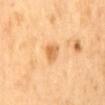Recorded during total-body skin imaging; not selected for excision or biopsy. A male patient in their mid- to late 60s. The lesion is on the mid back. About 2.5 mm across. Cropped from a whole-body photographic skin survey; the tile spans about 15 mm. The tile uses cross-polarized illumination.